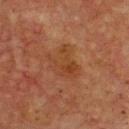Clinical impression:
The lesion was tiled from a total-body skin photograph and was not biopsied.
Background:
This image is a 15 mm lesion crop taken from a total-body photograph. Located on the front of the torso. A male subject approximately 75 years of age. The lesion-visualizer software estimated a lesion color around L≈33 a*≈20 b*≈29 in CIELAB and a normalized lesion–skin contrast near 5.5. The analysis additionally found border irregularity of about 5.5 on a 0–10 scale. It also reported an automated nevus-likeness rating near 0 out of 100 and a lesion-detection confidence of about 100/100. Measured at roughly 4.5 mm in maximum diameter. The tile uses cross-polarized illumination.